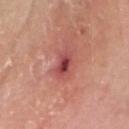<record>
<patient>
  <sex>male</sex>
  <age_approx>65</age_approx>
</patient>
<automated_metrics>
  <cielab_L>47</cielab_L>
  <cielab_a>33</cielab_a>
  <cielab_b>25</cielab_b>
  <vs_skin_darker_L>12.0</vs_skin_darker_L>
  <vs_skin_contrast_norm>9.0</vs_skin_contrast_norm>
  <border_irregularity_0_10>2.0</border_irregularity_0_10>
</automated_metrics>
<site>head or neck</site>
<lighting>white-light</lighting>
<image>
  <source>total-body photography crop</source>
  <field_of_view_mm>15</field_of_view_mm>
</image>
</record>